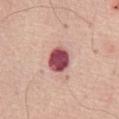The lesion was tiled from a total-body skin photograph and was not biopsied. On the abdomen. A male subject aged approximately 70. Cropped from a whole-body photographic skin survey; the tile spans about 15 mm. Approximately 3.5 mm at its widest.